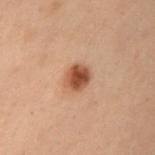Notes:
- follow-up — catalogued during a skin exam; not biopsied
- image source — ~15 mm tile from a whole-body skin photo
- automated lesion analysis — an outline eccentricity of about 0.5 (0 = round, 1 = elongated) and a shape-asymmetry score of about 0.15 (0 = symmetric); an automated nevus-likeness rating near 100 out of 100
- subject — female, in their mid-50s
- tile lighting — cross-polarized illumination
- anatomic site — the left upper arm